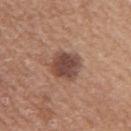Case summary:
* notes: total-body-photography surveillance lesion; no biopsy
* image source: ~15 mm crop, total-body skin-cancer survey
* lighting: white-light
* patient: female, aged 53–57
* TBP lesion metrics: an outline eccentricity of about 0.4 (0 = round, 1 = elongated) and two-axis asymmetry of about 0.15; a lesion color around L≈46 a*≈20 b*≈25 in CIELAB, roughly 13 lightness units darker than nearby skin, and a lesion-to-skin contrast of about 9.5 (normalized; higher = more distinct); internal color variation of about 4 on a 0–10 scale and peripheral color asymmetry of about 1; an automated nevus-likeness rating near 65 out of 100 and a lesion-detection confidence of about 100/100
* lesion diameter: about 3.5 mm
* site: the right upper arm